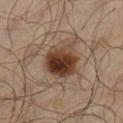Q: Was a biopsy performed?
A: catalogued during a skin exam; not biopsied
Q: How large is the lesion?
A: ≈4.5 mm
Q: What are the patient's age and sex?
A: male, approximately 65 years of age
Q: How was the tile lit?
A: cross-polarized illumination
Q: What is the anatomic site?
A: the left lower leg
Q: What is the imaging modality?
A: total-body-photography crop, ~15 mm field of view